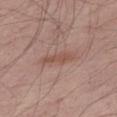Imaged during a routine full-body skin examination; the lesion was not biopsied and no histopathology is available.
A male patient aged approximately 55.
Longest diameter approximately 3.5 mm.
A roughly 15 mm field-of-view crop from a total-body skin photograph.
On the right thigh.